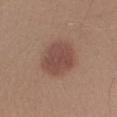This is a white-light tile. The total-body-photography lesion software estimated an average lesion color of about L≈47 a*≈20 b*≈25 (CIELAB), about 9 CIELAB-L* units darker than the surrounding skin, and a normalized border contrast of about 7. It also reported border irregularity of about 1.5 on a 0–10 scale, a color-variation rating of about 2.5/10, and a peripheral color-asymmetry measure near 0.5. The software also gave lesion-presence confidence of about 100/100. Longest diameter approximately 5 mm. The lesion is located on the left lower leg. A male patient aged 28–32. Cropped from a whole-body photographic skin survey; the tile spans about 15 mm.